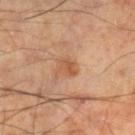Assessment: Part of a total-body skin-imaging series; this lesion was reviewed on a skin check and was not flagged for biopsy. Image and clinical context: A male patient, in their 70s. Located on the right lower leg. A roughly 15 mm field-of-view crop from a total-body skin photograph. Imaged with cross-polarized lighting. An algorithmic analysis of the crop reported an area of roughly 4 mm² and a shape-asymmetry score of about 0.25 (0 = symmetric). The software also gave border irregularity of about 2.5 on a 0–10 scale, a color-variation rating of about 3/10, and radial color variation of about 1. The lesion's longest dimension is about 2.5 mm.